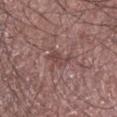Impression: This lesion was catalogued during total-body skin photography and was not selected for biopsy.